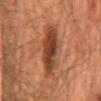Clinical impression: Captured during whole-body skin photography for melanoma surveillance; the lesion was not biopsied. Acquisition and patient details: A male subject aged 58 to 62. A lesion tile, about 15 mm wide, cut from a 3D total-body photograph. From the mid back.